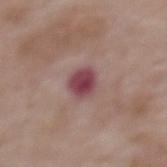This lesion was catalogued during total-body skin photography and was not selected for biopsy.
This is a white-light tile.
A 15 mm crop from a total-body photograph taken for skin-cancer surveillance.
A male subject, about 85 years old or older.
The lesion is located on the upper back.